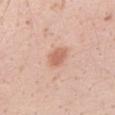{"biopsy_status": "not biopsied; imaged during a skin examination", "lighting": "white-light", "automated_metrics": {"area_mm2_approx": 4.5, "eccentricity": 0.7, "shape_asymmetry": 0.15, "cielab_L": 63, "cielab_a": 24, "cielab_b": 31, "vs_skin_darker_L": 10.0, "vs_skin_contrast_norm": 7.0}, "patient": {"sex": "female", "age_approx": 20}, "lesion_size": {"long_diameter_mm_approx": 3.0}, "image": {"source": "total-body photography crop", "field_of_view_mm": 15}, "site": "left forearm"}